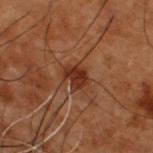The lesion was tiled from a total-body skin photograph and was not biopsied.
On the upper back.
The recorded lesion diameter is about 3 mm.
A male subject, approximately 50 years of age.
This is a cross-polarized tile.
A lesion tile, about 15 mm wide, cut from a 3D total-body photograph.